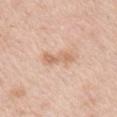Imaged during a routine full-body skin examination; the lesion was not biopsied and no histopathology is available. The total-body-photography lesion software estimated about 9 CIELAB-L* units darker than the surrounding skin and a normalized border contrast of about 6.5. The analysis additionally found a classifier nevus-likeness of about 0/100 and a lesion-detection confidence of about 100/100. A female patient, about 40 years old. A roughly 15 mm field-of-view crop from a total-body skin photograph. The lesion is located on the right upper arm. About 3.5 mm across. Captured under white-light illumination.